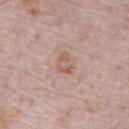| field | value |
|---|---|
| illumination | white-light |
| patient | male, aged approximately 70 |
| site | the abdomen |
| image source | 15 mm crop, total-body photography |
| size | ≈2.5 mm |